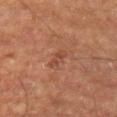About 3 mm across.
The lesion is on the left upper arm.
A male subject, about 75 years old.
This is a cross-polarized tile.
The total-body-photography lesion software estimated a footprint of about 3 mm² and a symmetry-axis asymmetry near 0.35. The software also gave about 6 CIELAB-L* units darker than the surrounding skin. The analysis additionally found a border-irregularity index near 4/10, a within-lesion color-variation index near 0/10, and radial color variation of about 0. And it measured a nevus-likeness score of about 0/100 and lesion-presence confidence of about 100/100.
A region of skin cropped from a whole-body photographic capture, roughly 15 mm wide.